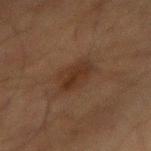<case>
<biopsy_status>not biopsied; imaged during a skin examination</biopsy_status>
</case>